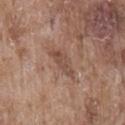Assessment:
No biopsy was performed on this lesion — it was imaged during a full skin examination and was not determined to be concerning.
Context:
A male patient aged approximately 75. The lesion is located on the back. Cropped from a whole-body photographic skin survey; the tile spans about 15 mm. Captured under white-light illumination.